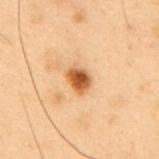Recorded during total-body skin imaging; not selected for excision or biopsy.
A 15 mm close-up tile from a total-body photography series done for melanoma screening.
About 3 mm across.
Located on the mid back.
Captured under cross-polarized illumination.
A male patient approximately 55 years of age.